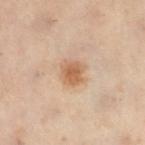<tbp_lesion>
<site>left leg</site>
<lighting>cross-polarized</lighting>
<lesion_size>
  <long_diameter_mm_approx>3.0</long_diameter_mm_approx>
</lesion_size>
<automated_metrics>
  <cielab_L>53</cielab_L>
  <cielab_a>17</cielab_a>
  <cielab_b>30</cielab_b>
  <vs_skin_darker_L>9.0</vs_skin_darker_L>
  <vs_skin_contrast_norm>7.5</vs_skin_contrast_norm>
  <color_variation_0_10>3.0</color_variation_0_10>
  <peripheral_color_asymmetry>1.0</peripheral_color_asymmetry>
</automated_metrics>
<image>
  <source>total-body photography crop</source>
  <field_of_view_mm>15</field_of_view_mm>
</image>
<patient>
  <sex>female</sex>
  <age_approx>60</age_approx>
</patient>
</tbp_lesion>